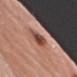notes: imaged on a skin check; not biopsied | image: 15 mm crop, total-body photography | diameter: about 3.5 mm | subject: female, aged 63–67 | location: the arm | lighting: white-light.